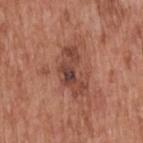{
  "biopsy_status": "not biopsied; imaged during a skin examination",
  "lighting": "white-light",
  "lesion_size": {
    "long_diameter_mm_approx": 5.5
  },
  "patient": {
    "sex": "male",
    "age_approx": 60
  },
  "image": {
    "source": "total-body photography crop",
    "field_of_view_mm": 15
  },
  "site": "upper back"
}